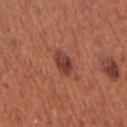About 3 mm across. Captured under white-light illumination. The lesion-visualizer software estimated an area of roughly 5 mm², a shape eccentricity near 0.7, and a shape-asymmetry score of about 0.2 (0 = symmetric). The software also gave an average lesion color of about L≈40 a*≈26 b*≈26 (CIELAB), a lesion–skin lightness drop of about 11, and a lesion-to-skin contrast of about 9.5 (normalized; higher = more distinct). The analysis additionally found border irregularity of about 1.5 on a 0–10 scale and a peripheral color-asymmetry measure near 1.5. The analysis additionally found a classifier nevus-likeness of about 85/100. The subject is a female aged 63 to 67. Located on the right upper arm. A close-up tile cropped from a whole-body skin photograph, about 15 mm across.